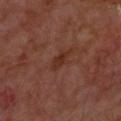From the upper back.
Imaged with cross-polarized lighting.
About 3 mm across.
A lesion tile, about 15 mm wide, cut from a 3D total-body photograph.
The subject is a male aged around 70.